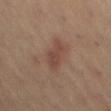notes = catalogued during a skin exam; not biopsied | illumination = cross-polarized | subject = female, about 40 years old | location = the left thigh | TBP lesion metrics = an average lesion color of about L≈44 a*≈19 b*≈25 (CIELAB) and a normalized lesion–skin contrast near 6; a border-irregularity index near 3.5/10 and a color-variation rating of about 2.5/10; a nevus-likeness score of about 25/100 | imaging modality = total-body-photography crop, ~15 mm field of view | lesion diameter = about 4.5 mm.